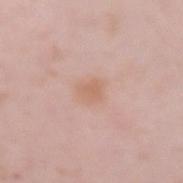Case summary:
- workup — imaged on a skin check; not biopsied
- location — the left forearm
- image-analysis metrics — a shape eccentricity near 0.45; a color-variation rating of about 1.5/10 and peripheral color asymmetry of about 0.5; a classifier nevus-likeness of about 15/100 and a lesion-detection confidence of about 100/100
- image source — ~15 mm crop, total-body skin-cancer survey
- diameter — about 2.5 mm
- patient — female, aged around 40
- illumination — white-light illumination